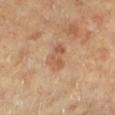  biopsy_status: not biopsied; imaged during a skin examination
  site: left lower leg
  image:
    source: total-body photography crop
    field_of_view_mm: 15
  patient:
    sex: female
    age_approx: 60
  lighting: cross-polarized
  lesion_size:
    long_diameter_mm_approx: 3.5
  automated_metrics:
    eccentricity: 0.75
    shape_asymmetry: 0.35
    nevus_likeness_0_100: 0
    lesion_detection_confidence_0_100: 100